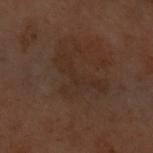Imaged during a routine full-body skin examination; the lesion was not biopsied and no histopathology is available. Measured at roughly 6 mm in maximum diameter. A female patient aged 58–62. A 15 mm close-up tile from a total-body photography series done for melanoma screening. Located on the front of the torso.